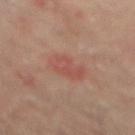Part of a total-body skin-imaging series; this lesion was reviewed on a skin check and was not flagged for biopsy. Captured under cross-polarized illumination. A male patient aged 63–67. On the mid back. The total-body-photography lesion software estimated a classifier nevus-likeness of about 0/100 and lesion-presence confidence of about 100/100. A 15 mm close-up tile from a total-body photography series done for melanoma screening. Approximately 3.5 mm at its widest.